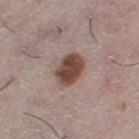Assessment:
Captured during whole-body skin photography for melanoma surveillance; the lesion was not biopsied.